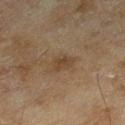Assessment: This lesion was catalogued during total-body skin photography and was not selected for biopsy. Clinical summary: Imaged with cross-polarized lighting. From the right lower leg. A female subject in their 60s. A close-up tile cropped from a whole-body skin photograph, about 15 mm across. Longest diameter approximately 2.5 mm.